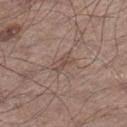{
  "lighting": "white-light",
  "lesion_size": {
    "long_diameter_mm_approx": 3.0
  },
  "patient": {
    "sex": "male",
    "age_approx": 55
  },
  "image": {
    "source": "total-body photography crop",
    "field_of_view_mm": 15
  },
  "site": "right lower leg"
}